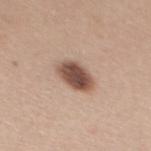Assessment:
Captured during whole-body skin photography for melanoma surveillance; the lesion was not biopsied.
Context:
The total-body-photography lesion software estimated an average lesion color of about L≈51 a*≈18 b*≈26 (CIELAB), roughly 17 lightness units darker than nearby skin, and a normalized lesion–skin contrast near 11. It also reported an automated nevus-likeness rating near 95 out of 100 and a lesion-detection confidence of about 100/100. This is a white-light tile. A region of skin cropped from a whole-body photographic capture, roughly 15 mm wide. The subject is a female in their 40s. From the upper back.